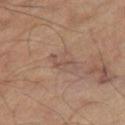Impression: The lesion was tiled from a total-body skin photograph and was not biopsied. Clinical summary: The lesion is on the right thigh. A male patient aged around 65. Longest diameter approximately 3.5 mm. This image is a 15 mm lesion crop taken from a total-body photograph.